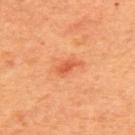Impression:
The lesion was tiled from a total-body skin photograph and was not biopsied.
Context:
The lesion-visualizer software estimated a mean CIELAB color near L≈60 a*≈35 b*≈44, a lesion–skin lightness drop of about 10, and a normalized lesion–skin contrast near 6.5. And it measured a border-irregularity index near 3.5/10, internal color variation of about 1 on a 0–10 scale, and peripheral color asymmetry of about 0.5. And it measured an automated nevus-likeness rating near 70 out of 100 and lesion-presence confidence of about 100/100. The lesion is on the back. A female patient, roughly 40 years of age. This is a cross-polarized tile. A close-up tile cropped from a whole-body skin photograph, about 15 mm across.